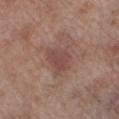No biopsy was performed on this lesion — it was imaged during a full skin examination and was not determined to be concerning.
A 15 mm crop from a total-body photograph taken for skin-cancer surveillance.
The patient is a male roughly 70 years of age.
The lesion's longest dimension is about 3.5 mm.
The lesion-visualizer software estimated a mean CIELAB color near L≈47 a*≈21 b*≈23 and roughly 7 lightness units darker than nearby skin. And it measured a color-variation rating of about 2.5/10 and peripheral color asymmetry of about 1.
From the leg.
The tile uses white-light illumination.